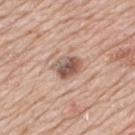Assessment:
Imaged during a routine full-body skin examination; the lesion was not biopsied and no histopathology is available.
Clinical summary:
A male patient, aged 78–82. A 15 mm close-up tile from a total-body photography series done for melanoma screening. Longest diameter approximately 3.5 mm. Located on the mid back. Imaged with white-light lighting.